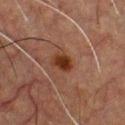Impression:
Part of a total-body skin-imaging series; this lesion was reviewed on a skin check and was not flagged for biopsy.
Image and clinical context:
The lesion is on the chest. Cropped from a total-body skin-imaging series; the visible field is about 15 mm. This is a cross-polarized tile. A male subject aged 48 to 52. An algorithmic analysis of the crop reported about 9 CIELAB-L* units darker than the surrounding skin and a normalized border contrast of about 10.5. The recorded lesion diameter is about 3 mm.